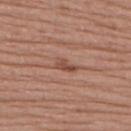{
  "biopsy_status": "not biopsied; imaged during a skin examination",
  "automated_metrics": {
    "cielab_L": 49,
    "cielab_a": 23,
    "cielab_b": 28,
    "vs_skin_darker_L": 9.0,
    "vs_skin_contrast_norm": 7.0,
    "border_irregularity_0_10": 4.0,
    "peripheral_color_asymmetry": 0.0
  },
  "site": "upper back",
  "lighting": "white-light",
  "lesion_size": {
    "long_diameter_mm_approx": 3.0
  },
  "patient": {
    "sex": "female",
    "age_approx": 55
  },
  "image": {
    "source": "total-body photography crop",
    "field_of_view_mm": 15
  }
}